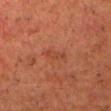This lesion was catalogued during total-body skin photography and was not selected for biopsy.
From the head or neck.
A region of skin cropped from a whole-body photographic capture, roughly 15 mm wide.
An algorithmic analysis of the crop reported a footprint of about 3 mm², an outline eccentricity of about 0.9 (0 = round, 1 = elongated), and a symmetry-axis asymmetry near 0.2. The software also gave border irregularity of about 3 on a 0–10 scale, a color-variation rating of about 0/10, and peripheral color asymmetry of about 0.
A male patient in their mid-60s.
Imaged with cross-polarized lighting.
Approximately 3 mm at its widest.